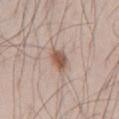biopsy status: no biopsy performed (imaged during a skin exam) | illumination: white-light illumination | body site: the abdomen | imaging modality: ~15 mm tile from a whole-body skin photo | patient: male, about 45 years old | automated lesion analysis: an outline eccentricity of about 0.7 (0 = round, 1 = elongated) and two-axis asymmetry of about 0.25; a mean CIELAB color near L≈55 a*≈18 b*≈26, roughly 12 lightness units darker than nearby skin, and a normalized border contrast of about 8.5; an automated nevus-likeness rating near 95 out of 100 and a lesion-detection confidence of about 100/100 | lesion diameter: ≈3.5 mm.